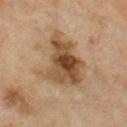Assessment: The lesion was photographed on a routine skin check and not biopsied; there is no pathology result. Acquisition and patient details: The patient is a female approximately 55 years of age. Automated image analysis of the tile measured an automated nevus-likeness rating near 55 out of 100 and a lesion-detection confidence of about 100/100. Located on the leg. A close-up tile cropped from a whole-body skin photograph, about 15 mm across. Imaged with cross-polarized lighting.